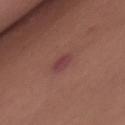• notes — catalogued during a skin exam; not biopsied
• location — the chest
• patient — female, aged around 50
• acquisition — 15 mm crop, total-body photography
• image-analysis metrics — a footprint of about 3 mm²; a classifier nevus-likeness of about 5/100 and a detector confidence of about 100 out of 100 that the crop contains a lesion